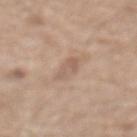Findings:
- workup · imaged on a skin check; not biopsied
- illumination · white-light
- site · the abdomen
- TBP lesion metrics · an area of roughly 4.5 mm²; a border-irregularity rating of about 3/10, a color-variation rating of about 2/10, and a peripheral color-asymmetry measure near 0.5
- image · total-body-photography crop, ~15 mm field of view
- size · ~3.5 mm (longest diameter)
- subject · male, aged 68 to 72